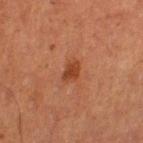This lesion was catalogued during total-body skin photography and was not selected for biopsy. Located on the right thigh. A male subject aged 63 to 67. The tile uses cross-polarized illumination. An algorithmic analysis of the crop reported a mean CIELAB color near L≈33 a*≈23 b*≈30, roughly 8 lightness units darker than nearby skin, and a lesion-to-skin contrast of about 8 (normalized; higher = more distinct). The software also gave a lesion-detection confidence of about 100/100. Cropped from a total-body skin-imaging series; the visible field is about 15 mm. The recorded lesion diameter is about 2.5 mm.